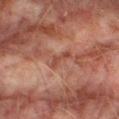{"biopsy_status": "not biopsied; imaged during a skin examination", "patient": {"sex": "male", "age_approx": 75}, "lighting": "cross-polarized", "automated_metrics": {"area_mm2_approx": 3.0, "eccentricity": 0.95, "shape_asymmetry": 0.55, "cielab_L": 38, "cielab_a": 22, "cielab_b": 25, "vs_skin_darker_L": 6.0, "vs_skin_contrast_norm": 6.0, "nevus_likeness_0_100": 0}, "lesion_size": {"long_diameter_mm_approx": 3.5}, "site": "right thigh", "image": {"source": "total-body photography crop", "field_of_view_mm": 15}}